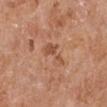<record>
<biopsy_status>not biopsied; imaged during a skin examination</biopsy_status>
<lighting>white-light</lighting>
<image>
  <source>total-body photography crop</source>
  <field_of_view_mm>15</field_of_view_mm>
</image>
<patient>
  <sex>male</sex>
  <age_approx>65</age_approx>
</patient>
<site>right upper arm</site>
<lesion_size>
  <long_diameter_mm_approx>4.0</long_diameter_mm_approx>
</lesion_size>
</record>